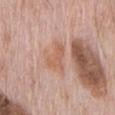Captured during whole-body skin photography for melanoma surveillance; the lesion was not biopsied. The subject is a male approximately 70 years of age. The tile uses white-light illumination. A 15 mm crop from a total-body photograph taken for skin-cancer surveillance. From the front of the torso. Measured at roughly 3 mm in maximum diameter. Automated tile analysis of the lesion measured a lesion area of about 6 mm², an eccentricity of roughly 0.75, and a symmetry-axis asymmetry near 0.45. The analysis additionally found about 7 CIELAB-L* units darker than the surrounding skin and a normalized lesion–skin contrast near 6.